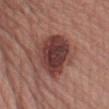{"site": "left upper arm", "patient": {"sex": "male", "age_approx": 75}, "automated_metrics": {"area_mm2_approx": 22.0, "eccentricity": 0.8, "shape_asymmetry": 0.15, "cielab_L": 39, "cielab_a": 23, "cielab_b": 22, "vs_skin_darker_L": 14.0, "vs_skin_contrast_norm": 11.0, "border_irregularity_0_10": 2.5, "peripheral_color_asymmetry": 1.5, "nevus_likeness_0_100": 90}, "image": {"source": "total-body photography crop", "field_of_view_mm": 15}}